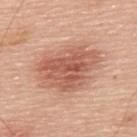Acquisition and patient details: Measured at roughly 7.5 mm in maximum diameter. A 15 mm close-up extracted from a 3D total-body photography capture. A male subject roughly 70 years of age. Located on the upper back. Captured under white-light illumination.